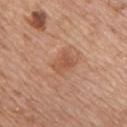  biopsy_status: not biopsied; imaged during a skin examination
  patient:
    sex: male
    age_approx: 60
  image:
    source: total-body photography crop
    field_of_view_mm: 15
  site: chest